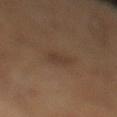Assessment: This lesion was catalogued during total-body skin photography and was not selected for biopsy. Background: The lesion is on the leg. The subject is a male about 45 years old. This image is a 15 mm lesion crop taken from a total-body photograph. The lesion's longest dimension is about 2.5 mm.